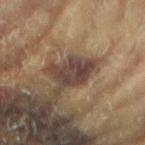This lesion was catalogued during total-body skin photography and was not selected for biopsy. The lesion is located on the left arm. A female subject roughly 80 years of age. Cropped from a total-body skin-imaging series; the visible field is about 15 mm.